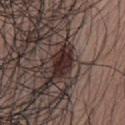Clinical impression: This lesion was catalogued during total-body skin photography and was not selected for biopsy. Image and clinical context: A male patient approximately 35 years of age. The lesion is on the chest. A 15 mm close-up extracted from a 3D total-body photography capture.